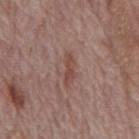Q: Was a biopsy performed?
A: total-body-photography surveillance lesion; no biopsy
Q: How was this image acquired?
A: total-body-photography crop, ~15 mm field of view
Q: What are the patient's age and sex?
A: male, aged 68–72
Q: What is the anatomic site?
A: the back